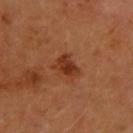Q: Is there a histopathology result?
A: total-body-photography surveillance lesion; no biopsy
Q: Lesion size?
A: ~3 mm (longest diameter)
Q: What is the anatomic site?
A: the left forearm
Q: What lighting was used for the tile?
A: cross-polarized
Q: What kind of image is this?
A: 15 mm crop, total-body photography
Q: Who is the patient?
A: female, aged approximately 70